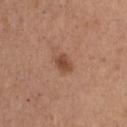Impression: Recorded during total-body skin imaging; not selected for excision or biopsy. Image and clinical context: The recorded lesion diameter is about 2.5 mm. A close-up tile cropped from a whole-body skin photograph, about 15 mm across. On the chest. A male subject, aged approximately 65.